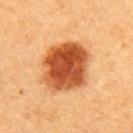Impression:
Captured during whole-body skin photography for melanoma surveillance; the lesion was not biopsied.
Acquisition and patient details:
A female patient, aged 63 to 67. On the upper back. Imaged with cross-polarized lighting. Measured at roughly 6 mm in maximum diameter. This image is a 15 mm lesion crop taken from a total-body photograph. The total-body-photography lesion software estimated a lesion color around L≈52 a*≈31 b*≈43 in CIELAB and about 20 CIELAB-L* units darker than the surrounding skin. The analysis additionally found a border-irregularity index near 1.5/10, a within-lesion color-variation index near 6.5/10, and radial color variation of about 2. And it measured an automated nevus-likeness rating near 100 out of 100 and a detector confidence of about 100 out of 100 that the crop contains a lesion.